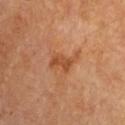biopsy_status: not biopsied; imaged during a skin examination
patient:
  sex: male
  age_approx: 85+
lesion_size:
  long_diameter_mm_approx: 3.5
lighting: cross-polarized
image:
  source: total-body photography crop
  field_of_view_mm: 15
site: chest